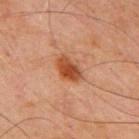The lesion's longest dimension is about 3.5 mm.
The tile uses cross-polarized illumination.
On the front of the torso.
A male patient about 70 years old.
A lesion tile, about 15 mm wide, cut from a 3D total-body photograph.